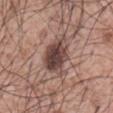Assessment:
Imaged during a routine full-body skin examination; the lesion was not biopsied and no histopathology is available.
Clinical summary:
Cropped from a total-body skin-imaging series; the visible field is about 15 mm. A male patient, aged around 60. Captured under white-light illumination. About 5.5 mm across. Located on the abdomen.